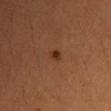Recorded during total-body skin imaging; not selected for excision or biopsy.
Measured at roughly 2 mm in maximum diameter.
A female patient aged 38 to 42.
The lesion is located on the left upper arm.
A 15 mm close-up extracted from a 3D total-body photography capture.
Automated image analysis of the tile measured roughly 9 lightness units darker than nearby skin and a lesion-to-skin contrast of about 8 (normalized; higher = more distinct). And it measured a border-irregularity index near 2.5/10, a within-lesion color-variation index near 2/10, and radial color variation of about 0.5.
Captured under cross-polarized illumination.